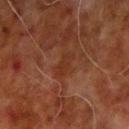follow-up = total-body-photography surveillance lesion; no biopsy
image = ~15 mm tile from a whole-body skin photo
anatomic site = the right upper arm
patient = male, aged 68 to 72
diameter = ≈3 mm